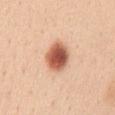notes=no biopsy performed (imaged during a skin exam).